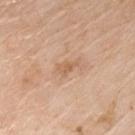Q: Is there a histopathology result?
A: catalogued during a skin exam; not biopsied
Q: How was the tile lit?
A: white-light illumination
Q: How large is the lesion?
A: ~2.5 mm (longest diameter)
Q: How was this image acquired?
A: ~15 mm crop, total-body skin-cancer survey
Q: Automated lesion metrics?
A: an outline eccentricity of about 0.85 (0 = round, 1 = elongated) and a symmetry-axis asymmetry near 0.4; a mean CIELAB color near L≈61 a*≈20 b*≈34, roughly 8 lightness units darker than nearby skin, and a normalized border contrast of about 6
Q: Lesion location?
A: the chest
Q: What are the patient's age and sex?
A: male, about 80 years old